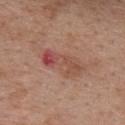Imaged during a routine full-body skin examination; the lesion was not biopsied and no histopathology is available. Located on the upper back. About 5.5 mm across. An algorithmic analysis of the crop reported a lesion color around L≈49 a*≈25 b*≈27 in CIELAB, a lesion–skin lightness drop of about 9, and a normalized border contrast of about 6.5. And it measured border irregularity of about 5 on a 0–10 scale. And it measured a classifier nevus-likeness of about 0/100 and a lesion-detection confidence of about 100/100. The subject is a female aged approximately 40. This image is a 15 mm lesion crop taken from a total-body photograph.